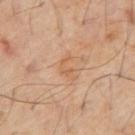  lesion_size:
    long_diameter_mm_approx: 3.0
  lighting: cross-polarized
  patient:
    sex: male
    age_approx: 60
  automated_metrics:
    area_mm2_approx: 4.0
    eccentricity: 0.8
    shape_asymmetry: 0.6
    cielab_L: 55
    cielab_a: 20
    cielab_b: 33
    vs_skin_darker_L: 6.0
    vs_skin_contrast_norm: 5.0
    nevus_likeness_0_100: 0
    lesion_detection_confidence_0_100: 100
  image:
    source: total-body photography crop
    field_of_view_mm: 15
  site: abdomen